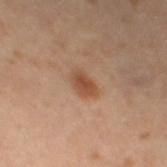About 3 mm across. The lesion is on the left thigh. A male subject, roughly 65 years of age. A lesion tile, about 15 mm wide, cut from a 3D total-body photograph.